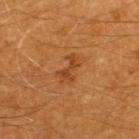Clinical impression: Imaged during a routine full-body skin examination; the lesion was not biopsied and no histopathology is available. Background: The tile uses cross-polarized illumination. On the back. Approximately 3.5 mm at its widest. A 15 mm close-up tile from a total-body photography series done for melanoma screening. A male patient, aged around 60.